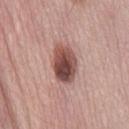This lesion was catalogued during total-body skin photography and was not selected for biopsy.
From the mid back.
A 15 mm crop from a total-body photograph taken for skin-cancer surveillance.
A male subject aged approximately 65.